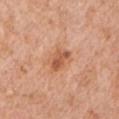{"biopsy_status": "not biopsied; imaged during a skin examination", "lighting": "white-light", "patient": {"sex": "male", "age_approx": 60}, "image": {"source": "total-body photography crop", "field_of_view_mm": 15}, "site": "left upper arm"}